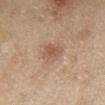Impression:
Captured during whole-body skin photography for melanoma surveillance; the lesion was not biopsied.
Clinical summary:
Imaged with cross-polarized lighting. A close-up tile cropped from a whole-body skin photograph, about 15 mm across. The lesion is on the left lower leg. A female subject approximately 60 years of age.